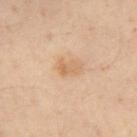biopsy_status: not biopsied; imaged during a skin examination
lesion_size:
  long_diameter_mm_approx: 2.5
image:
  source: total-body photography crop
  field_of_view_mm: 15
site: chest
lighting: cross-polarized
automated_metrics:
  cielab_L: 53
  cielab_a: 16
  cielab_b: 31
  vs_skin_darker_L: 7.0
  vs_skin_contrast_norm: 5.5
  nevus_likeness_0_100: 25
  lesion_detection_confidence_0_100: 100
patient:
  sex: female
  age_approx: 55